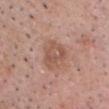The lesion was photographed on a routine skin check and not biopsied; there is no pathology result. On the head or neck. The subject is a male aged 53–57. The lesion-visualizer software estimated an area of roughly 8.5 mm² and a shape-asymmetry score of about 0.3 (0 = symmetric). And it measured a lesion–skin lightness drop of about 8 and a normalized border contrast of about 6. And it measured a border-irregularity rating of about 3.5/10, a within-lesion color-variation index near 4/10, and radial color variation of about 1. This image is a 15 mm lesion crop taken from a total-body photograph.